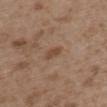The total-body-photography lesion software estimated a lesion area of about 2.5 mm², an outline eccentricity of about 0.9 (0 = round, 1 = elongated), and two-axis asymmetry of about 0.35. The analysis additionally found an average lesion color of about L≈46 a*≈18 b*≈29 (CIELAB), about 8 CIELAB-L* units darker than the surrounding skin, and a normalized lesion–skin contrast near 7.
A close-up tile cropped from a whole-body skin photograph, about 15 mm across.
The lesion is on the upper back.
Captured under white-light illumination.
The lesion's longest dimension is about 2.5 mm.
The subject is a female aged 33 to 37.